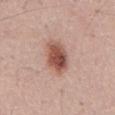Q: Lesion size?
A: about 4.5 mm
Q: Where on the body is the lesion?
A: the front of the torso
Q: How was this image acquired?
A: ~15 mm tile from a whole-body skin photo
Q: Patient demographics?
A: male, aged approximately 55